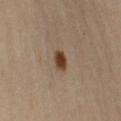workup — catalogued during a skin exam; not biopsied | lesion diameter — ≈3 mm | site — the arm | patient — male, aged around 55 | acquisition — ~15 mm crop, total-body skin-cancer survey | illumination — cross-polarized.